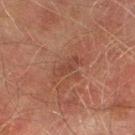Impression: Imaged during a routine full-body skin examination; the lesion was not biopsied and no histopathology is available. Context: A male patient about 75 years old. A 15 mm crop from a total-body photograph taken for skin-cancer surveillance. The lesion is located on the right thigh.